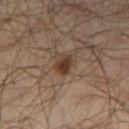Findings:
• workup — no biopsy performed (imaged during a skin exam)
• acquisition — 15 mm crop, total-body photography
• patient — male, in their mid- to late 60s
• site — the right thigh
• automated metrics — a border-irregularity index near 1.5/10 and a peripheral color-asymmetry measure near 1; a nevus-likeness score of about 95/100 and lesion-presence confidence of about 100/100
• lesion size — about 2.5 mm